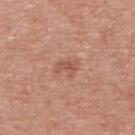Q: Is there a histopathology result?
A: catalogued during a skin exam; not biopsied
Q: Lesion size?
A: ~2.5 mm (longest diameter)
Q: What are the patient's age and sex?
A: female, in their 70s
Q: What is the imaging modality?
A: ~15 mm crop, total-body skin-cancer survey
Q: What lighting was used for the tile?
A: white-light
Q: What did automated image analysis measure?
A: an area of roughly 4 mm² and an eccentricity of roughly 0.75; a lesion color around L≈54 a*≈24 b*≈29 in CIELAB; border irregularity of about 5 on a 0–10 scale and peripheral color asymmetry of about 1; an automated nevus-likeness rating near 5 out of 100 and a detector confidence of about 100 out of 100 that the crop contains a lesion
Q: Where on the body is the lesion?
A: the upper back